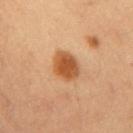Clinical impression: Captured during whole-body skin photography for melanoma surveillance; the lesion was not biopsied. Background: Imaged with cross-polarized lighting. The lesion is located on the arm. An algorithmic analysis of the crop reported border irregularity of about 1.5 on a 0–10 scale and peripheral color asymmetry of about 1. And it measured an automated nevus-likeness rating near 100 out of 100 and a lesion-detection confidence of about 100/100. About 3.5 mm across. A 15 mm close-up extracted from a 3D total-body photography capture. The subject is a female about 35 years old.